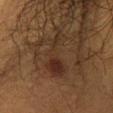biopsy status = imaged on a skin check; not biopsied | diameter = ≈6.5 mm | image source = ~15 mm tile from a whole-body skin photo | automated metrics = border irregularity of about 9 on a 0–10 scale, a within-lesion color-variation index near 3/10, and peripheral color asymmetry of about 0.5; a classifier nevus-likeness of about 85/100 | site = the front of the torso | subject = male, aged 38–42 | illumination = cross-polarized illumination.